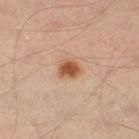workup=imaged on a skin check; not biopsied | body site=the left thigh | lighting=cross-polarized illumination | image=total-body-photography crop, ~15 mm field of view | size=~2.5 mm (longest diameter) | patient=aged around 55.